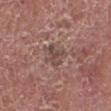Clinical impression: Recorded during total-body skin imaging; not selected for excision or biopsy. Context: The lesion's longest dimension is about 3 mm. Located on the leg. An algorithmic analysis of the crop reported an outline eccentricity of about 0.65 (0 = round, 1 = elongated). The analysis additionally found a lesion color around L≈46 a*≈18 b*≈21 in CIELAB and a normalized lesion–skin contrast near 6.5. It also reported a color-variation rating of about 3.5/10 and radial color variation of about 1. A 15 mm crop from a total-body photograph taken for skin-cancer surveillance. The tile uses white-light illumination. The subject is a male aged approximately 80.